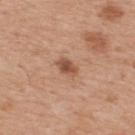The lesion was tiled from a total-body skin photograph and was not biopsied. From the upper back. A male patient aged 63–67. A lesion tile, about 15 mm wide, cut from a 3D total-body photograph.